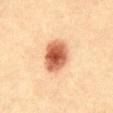The lesion was tiled from a total-body skin photograph and was not biopsied. The total-body-photography lesion software estimated an area of roughly 12 mm², an eccentricity of roughly 0.65, and a shape-asymmetry score of about 0.15 (0 = symmetric). The software also gave a lesion–skin lightness drop of about 18 and a normalized lesion–skin contrast near 11.5. And it measured a classifier nevus-likeness of about 100/100 and lesion-presence confidence of about 100/100. Longest diameter approximately 4 mm. Imaged with cross-polarized lighting. Cropped from a whole-body photographic skin survey; the tile spans about 15 mm. The lesion is on the front of the torso. A male patient in their mid-30s.